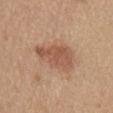The lesion was tiled from a total-body skin photograph and was not biopsied. The patient is a female aged approximately 55. On the mid back. This image is a 15 mm lesion crop taken from a total-body photograph. This is a white-light tile.